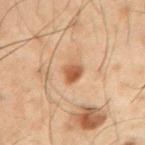Q: Was this lesion biopsied?
A: no biopsy performed (imaged during a skin exam)
Q: What is the imaging modality?
A: total-body-photography crop, ~15 mm field of view
Q: What is the anatomic site?
A: the right upper arm
Q: Patient demographics?
A: male, about 50 years old
Q: What lighting was used for the tile?
A: cross-polarized illumination